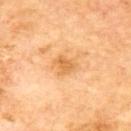biopsy_status: not biopsied; imaged during a skin examination
site: back
patient:
  sex: male
  age_approx: 70
lesion_size:
  long_diameter_mm_approx: 3.0
automated_metrics:
  cielab_L: 66
  cielab_a: 23
  cielab_b: 46
  vs_skin_contrast_norm: 6.5
lighting: cross-polarized
image:
  source: total-body photography crop
  field_of_view_mm: 15